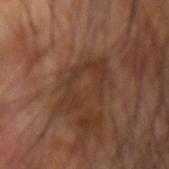| feature | finding |
|---|---|
| follow-up | no biopsy performed (imaged during a skin exam) |
| body site | the left arm |
| lesion size | ~5.5 mm (longest diameter) |
| patient | male, aged around 60 |
| acquisition | ~15 mm crop, total-body skin-cancer survey |